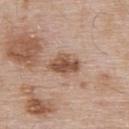From the upper back. A 15 mm close-up extracted from a 3D total-body photography capture. The recorded lesion diameter is about 4 mm. A male subject, aged 53–57. Imaged with white-light lighting.